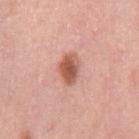Clinical impression:
The lesion was photographed on a routine skin check and not biopsied; there is no pathology result.